On the head or neck; a roughly 15 mm field-of-view crop from a total-body skin photograph; a male subject, about 70 years old:
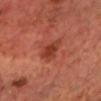Automated tile analysis of the lesion measured a footprint of about 6.5 mm², an eccentricity of roughly 0.55, and two-axis asymmetry of about 0.3. And it measured an average lesion color of about L≈38 a*≈30 b*≈31 (CIELAB), roughly 9 lightness units darker than nearby skin, and a lesion-to-skin contrast of about 7.5 (normalized; higher = more distinct). It also reported a border-irregularity index near 3/10, a color-variation rating of about 3/10, and radial color variation of about 1. And it measured a nevus-likeness score of about 55/100. The lesion's longest dimension is about 3.5 mm. Histopathological examination showed a nodular basal cell carcinoma.